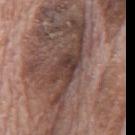  biopsy_status: not biopsied; imaged during a skin examination